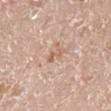No biopsy was performed on this lesion — it was imaged during a full skin examination and was not determined to be concerning. The lesion is on the right leg. A 15 mm crop from a total-body photograph taken for skin-cancer surveillance. This is a white-light tile. A male patient roughly 70 years of age.